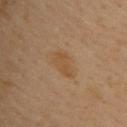This lesion was catalogued during total-body skin photography and was not selected for biopsy. Located on the upper back. A 15 mm crop from a total-body photograph taken for skin-cancer surveillance. The patient is a male in their 40s.